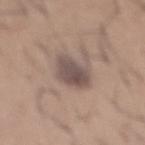| feature | finding |
|---|---|
| subject | male, aged around 65 |
| diameter | about 4 mm |
| imaging modality | 15 mm crop, total-body photography |
| body site | the mid back |
| tile lighting | white-light illumination |